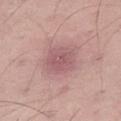image = ~15 mm crop, total-body skin-cancer survey | anatomic site = the leg | patient = male, aged around 55.